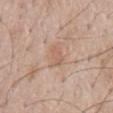Image and clinical context: A 15 mm close-up tile from a total-body photography series done for melanoma screening. Automated image analysis of the tile measured a mean CIELAB color near L≈60 a*≈19 b*≈29, about 7 CIELAB-L* units darker than the surrounding skin, and a normalized border contrast of about 5. The subject is a male aged around 60. Imaged with white-light lighting. Located on the mid back. About 3.5 mm across.